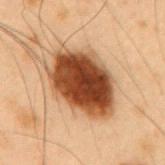The lesion was photographed on a routine skin check and not biopsied; there is no pathology result.